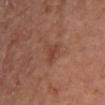Clinical impression: The lesion was photographed on a routine skin check and not biopsied; there is no pathology result. Acquisition and patient details: About 3 mm across. On the chest. A male subject, aged 53 to 57. A close-up tile cropped from a whole-body skin photograph, about 15 mm across. Automated image analysis of the tile measured an area of roughly 4.5 mm² and an eccentricity of roughly 0.65. The software also gave a lesion color around L≈43 a*≈23 b*≈28 in CIELAB and a lesion–skin lightness drop of about 6. The tile uses white-light illumination.